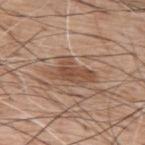Part of a total-body skin-imaging series; this lesion was reviewed on a skin check and was not flagged for biopsy.
From the upper back.
A 15 mm close-up extracted from a 3D total-body photography capture.
Automated tile analysis of the lesion measured an area of roughly 10 mm² and an outline eccentricity of about 0.85 (0 = round, 1 = elongated). And it measured an average lesion color of about L≈50 a*≈19 b*≈29 (CIELAB), roughly 10 lightness units darker than nearby skin, and a normalized border contrast of about 7.5.
A male patient, aged around 60.